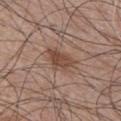Q: Was this lesion biopsied?
A: imaged on a skin check; not biopsied
Q: Where on the body is the lesion?
A: the chest
Q: How was this image acquired?
A: total-body-photography crop, ~15 mm field of view
Q: What lighting was used for the tile?
A: white-light
Q: Lesion size?
A: ~4.5 mm (longest diameter)
Q: Patient demographics?
A: male, aged around 50